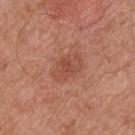anatomic site: the upper back
lighting: white-light
lesion size: about 4 mm
automated lesion analysis: an eccentricity of roughly 0.8 and two-axis asymmetry of about 0.15; an average lesion color of about L≈50 a*≈26 b*≈31 (CIELAB), roughly 7 lightness units darker than nearby skin, and a normalized border contrast of about 5.5; a border-irregularity rating of about 2/10 and peripheral color asymmetry of about 1
image: 15 mm crop, total-body photography
subject: male, aged around 55